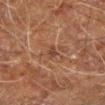Assessment: Imaged during a routine full-body skin examination; the lesion was not biopsied and no histopathology is available. Background: From the arm. Longest diameter approximately 2.5 mm. A close-up tile cropped from a whole-body skin photograph, about 15 mm across. The patient is a male aged around 60. The lesion-visualizer software estimated a lesion area of about 2 mm², an outline eccentricity of about 0.9 (0 = round, 1 = elongated), and two-axis asymmetry of about 0.4. The analysis additionally found an average lesion color of about L≈32 a*≈17 b*≈23 (CIELAB), about 6 CIELAB-L* units darker than the surrounding skin, and a normalized border contrast of about 6. It also reported border irregularity of about 4 on a 0–10 scale, a color-variation rating of about 0/10, and a peripheral color-asymmetry measure near 0. Imaged with cross-polarized lighting.